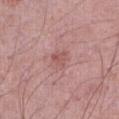biopsy status: total-body-photography surveillance lesion; no biopsy
body site: the front of the torso
size: about 3 mm
subject: male, roughly 70 years of age
automated lesion analysis: a footprint of about 5 mm² and an outline eccentricity of about 0.6 (0 = round, 1 = elongated); a nevus-likeness score of about 0/100 and lesion-presence confidence of about 100/100
tile lighting: white-light illumination
imaging modality: total-body-photography crop, ~15 mm field of view